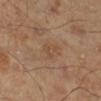Q: Was this lesion biopsied?
A: catalogued during a skin exam; not biopsied
Q: Patient demographics?
A: male, aged approximately 45
Q: Lesion location?
A: the leg
Q: How was this image acquired?
A: ~15 mm crop, total-body skin-cancer survey
Q: What lighting was used for the tile?
A: cross-polarized illumination